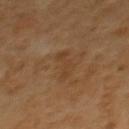<lesion>
<biopsy_status>not biopsied; imaged during a skin examination</biopsy_status>
<image>
  <source>total-body photography crop</source>
  <field_of_view_mm>15</field_of_view_mm>
</image>
<patient>
  <sex>female</sex>
  <age_approx>65</age_approx>
</patient>
<site>upper back</site>
</lesion>